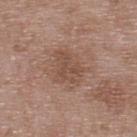  biopsy_status: not biopsied; imaged during a skin examination